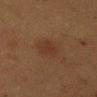biopsy status=imaged on a skin check; not biopsied | patient=female, aged 48 to 52 | tile lighting=cross-polarized illumination | anatomic site=the mid back | automated metrics=a lesion area of about 6.5 mm², a shape eccentricity near 0.75, and a symmetry-axis asymmetry near 0.2; lesion-presence confidence of about 100/100 | lesion size=~3.5 mm (longest diameter) | image source=total-body-photography crop, ~15 mm field of view.